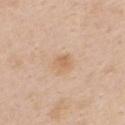<tbp_lesion>
  <biopsy_status>not biopsied; imaged during a skin examination</biopsy_status>
  <site>chest</site>
  <image>
    <source>total-body photography crop</source>
    <field_of_view_mm>15</field_of_view_mm>
  </image>
  <patient>
    <sex>female</sex>
    <age_approx>55</age_approx>
  </patient>
  <lighting>white-light</lighting>
</tbp_lesion>